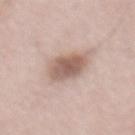| feature | finding |
|---|---|
| notes | imaged on a skin check; not biopsied |
| TBP lesion metrics | an outline eccentricity of about 0.45 (0 = round, 1 = elongated) and a symmetry-axis asymmetry near 0.15; a lesion color around L≈59 a*≈17 b*≈24 in CIELAB; internal color variation of about 3.5 on a 0–10 scale |
| site | the lower back |
| illumination | white-light |
| patient | male, roughly 50 years of age |
| imaging modality | total-body-photography crop, ~15 mm field of view |